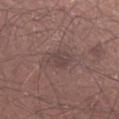Imaged during a routine full-body skin examination; the lesion was not biopsied and no histopathology is available.
The patient is a male aged around 30.
Automated tile analysis of the lesion measured an automated nevus-likeness rating near 0 out of 100 and lesion-presence confidence of about 75/100.
A lesion tile, about 15 mm wide, cut from a 3D total-body photograph.
The lesion's longest dimension is about 3.5 mm.
Located on the left forearm.